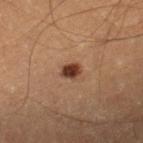biopsy status: total-body-photography surveillance lesion; no biopsy
image source: ~15 mm crop, total-body skin-cancer survey
automated lesion analysis: a mean CIELAB color near L≈36 a*≈21 b*≈28, a lesion–skin lightness drop of about 16, and a lesion-to-skin contrast of about 12.5 (normalized; higher = more distinct); a border-irregularity rating of about 1.5/10, internal color variation of about 3.5 on a 0–10 scale, and radial color variation of about 1.5; an automated nevus-likeness rating near 100 out of 100 and a lesion-detection confidence of about 100/100
subject: male, aged 18–22
lighting: cross-polarized
diameter: ≈2 mm
location: the right lower leg